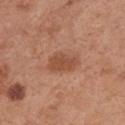biopsy_status: not biopsied; imaged during a skin examination
image:
  source: total-body photography crop
  field_of_view_mm: 15
site: chest
patient:
  sex: female
  age_approx: 65
lesion_size:
  long_diameter_mm_approx: 4.0
lighting: white-light